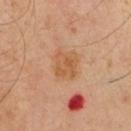Case summary:
- workup — total-body-photography surveillance lesion; no biopsy
- lesion size — ~3 mm (longest diameter)
- image-analysis metrics — a lesion color around L≈54 a*≈21 b*≈37 in CIELAB, a lesion–skin lightness drop of about 7, and a lesion-to-skin contrast of about 6.5 (normalized; higher = more distinct); a classifier nevus-likeness of about 25/100
- patient — male, about 65 years old
- location — the chest
- lighting — cross-polarized
- image — total-body-photography crop, ~15 mm field of view